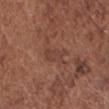  biopsy_status: not biopsied; imaged during a skin examination
  patient:
    sex: male
    age_approx: 75
  image:
    source: total-body photography crop
    field_of_view_mm: 15
  site: head or neck